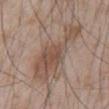{
  "biopsy_status": "not biopsied; imaged during a skin examination",
  "site": "abdomen",
  "image": {
    "source": "total-body photography crop",
    "field_of_view_mm": 15
  },
  "patient": {
    "sex": "male",
    "age_approx": 65
  }
}